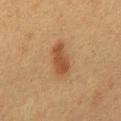The lesion was tiled from a total-body skin photograph and was not biopsied.
The lesion's longest dimension is about 4 mm.
A female patient roughly 50 years of age.
The lesion is located on the mid back.
Cropped from a whole-body photographic skin survey; the tile spans about 15 mm.
Captured under cross-polarized illumination.